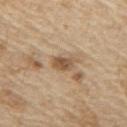<lesion>
<site>right upper arm</site>
<patient>
  <sex>male</sex>
  <age_approx>70</age_approx>
</patient>
<image>
  <source>total-body photography crop</source>
  <field_of_view_mm>15</field_of_view_mm>
</image>
<lesion_size>
  <long_diameter_mm_approx>2.5</long_diameter_mm_approx>
</lesion_size>
<automated_metrics>
  <area_mm2_approx>4.5</area_mm2_approx>
  <eccentricity>0.75</eccentricity>
  <shape_asymmetry>0.25</shape_asymmetry>
  <color_variation_0_10>4.5</color_variation_0_10>
  <peripheral_color_asymmetry>1.5</peripheral_color_asymmetry>
  <nevus_likeness_0_100>85</nevus_likeness_0_100>
  <lesion_detection_confidence_0_100>90</lesion_detection_confidence_0_100>
</automated_metrics>
</lesion>